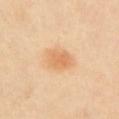Impression: The lesion was photographed on a routine skin check and not biopsied; there is no pathology result. Context: On the chest. This image is a 15 mm lesion crop taken from a total-body photograph. A female subject aged 38 to 42. Imaged with cross-polarized lighting.